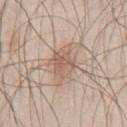Impression: The lesion was photographed on a routine skin check and not biopsied; there is no pathology result. Clinical summary: A male patient, aged 43 to 47. From the front of the torso. A lesion tile, about 15 mm wide, cut from a 3D total-body photograph. The tile uses white-light illumination. Longest diameter approximately 4 mm.